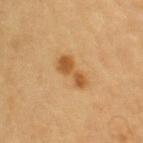Clinical impression:
Part of a total-body skin-imaging series; this lesion was reviewed on a skin check and was not flagged for biopsy.
Background:
A male patient approximately 85 years of age or older. Located on the arm. This is a cross-polarized tile. Cropped from a whole-body photographic skin survey; the tile spans about 15 mm. Longest diameter approximately 4.5 mm. An algorithmic analysis of the crop reported a lesion color around L≈47 a*≈19 b*≈38 in CIELAB, about 10 CIELAB-L* units darker than the surrounding skin, and a normalized lesion–skin contrast near 8. The software also gave a classifier nevus-likeness of about 95/100 and a detector confidence of about 100 out of 100 that the crop contains a lesion.